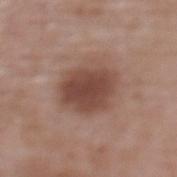follow-up = catalogued during a skin exam; not biopsied
illumination = white-light
patient = female, in their mid- to late 60s
lesion diameter = ~5.5 mm (longest diameter)
body site = the upper back
image source = ~15 mm tile from a whole-body skin photo